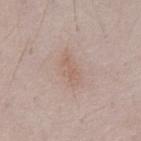biopsy status: imaged on a skin check; not biopsied | patient: male, aged 48 to 52 | image source: ~15 mm crop, total-body skin-cancer survey | TBP lesion metrics: a border-irregularity index near 3/10, a color-variation rating of about 2.5/10, and peripheral color asymmetry of about 1 | lesion diameter: about 4.5 mm | lighting: white-light illumination | body site: the front of the torso.